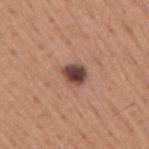workup: catalogued during a skin exam; not biopsied
acquisition: 15 mm crop, total-body photography
lighting: white-light illumination
subject: male, aged 43 to 47
diameter: about 3 mm
site: the right upper arm